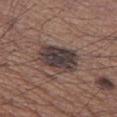Captured during whole-body skin photography for melanoma surveillance; the lesion was not biopsied.
Automated image analysis of the tile measured an area of roughly 16 mm² and a shape-asymmetry score of about 0.15 (0 = symmetric). It also reported a border-irregularity index near 2/10, a color-variation rating of about 5.5/10, and a peripheral color-asymmetry measure near 1.5. It also reported a detector confidence of about 100 out of 100 that the crop contains a lesion.
The tile uses white-light illumination.
A male patient about 65 years old.
A 15 mm crop from a total-body photograph taken for skin-cancer surveillance.
Approximately 5 mm at its widest.
Located on the left thigh.